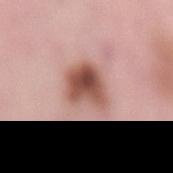<tbp_lesion>
<biopsy_status>not biopsied; imaged during a skin examination</biopsy_status>
<patient>
  <sex>male</sex>
  <age_approx>55</age_approx>
</patient>
<image>
  <source>total-body photography crop</source>
  <field_of_view_mm>15</field_of_view_mm>
</image>
<lesion_size>
  <long_diameter_mm_approx>4.5</long_diameter_mm_approx>
</lesion_size>
<site>lower back</site>
</tbp_lesion>